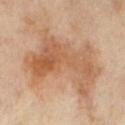{"biopsy_status": "not biopsied; imaged during a skin examination", "patient": {"sex": "female", "age_approx": 70}, "automated_metrics": {"cielab_L": 60, "cielab_a": 21, "cielab_b": 36, "vs_skin_darker_L": 10.0, "vs_skin_contrast_norm": 7.0, "border_irregularity_0_10": 7.5, "color_variation_0_10": 6.5}, "image": {"source": "total-body photography crop", "field_of_view_mm": 15}, "site": "leg", "lesion_size": {"long_diameter_mm_approx": 10.0}, "lighting": "cross-polarized"}